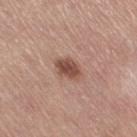Imaged during a routine full-body skin examination; the lesion was not biopsied and no histopathology is available. Measured at roughly 3 mm in maximum diameter. A lesion tile, about 15 mm wide, cut from a 3D total-body photograph. The subject is a female approximately 65 years of age. On the right thigh. Captured under white-light illumination.